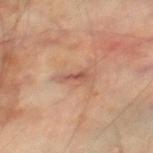{"biopsy_status": "not biopsied; imaged during a skin examination", "site": "right thigh", "patient": {"sex": "male", "age_approx": 70}, "image": {"source": "total-body photography crop", "field_of_view_mm": 15}, "lesion_size": {"long_diameter_mm_approx": 2.5}, "automated_metrics": {"area_mm2_approx": 2.5, "eccentricity": 0.9, "border_irregularity_0_10": 4.5}}